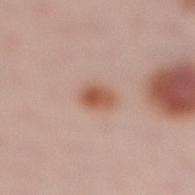notes = imaged on a skin check; not biopsied | body site = the right lower leg | imaging modality = ~15 mm crop, total-body skin-cancer survey | patient = female, roughly 25 years of age | lesion diameter = about 3 mm | automated metrics = a border-irregularity rating of about 2.5/10, internal color variation of about 4.5 on a 0–10 scale, and peripheral color asymmetry of about 1.5.